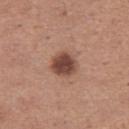This lesion was catalogued during total-body skin photography and was not selected for biopsy. The lesion is on the leg. A roughly 15 mm field-of-view crop from a total-body skin photograph. The lesion-visualizer software estimated a footprint of about 7.5 mm², an outline eccentricity of about 0.35 (0 = round, 1 = elongated), and a symmetry-axis asymmetry near 0.15. It also reported border irregularity of about 1 on a 0–10 scale, a color-variation rating of about 3/10, and peripheral color asymmetry of about 1. The analysis additionally found a nevus-likeness score of about 80/100 and lesion-presence confidence of about 100/100. The subject is a female aged approximately 30.